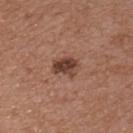Clinical impression:
Imaged during a routine full-body skin examination; the lesion was not biopsied and no histopathology is available.
Background:
Cropped from a total-body skin-imaging series; the visible field is about 15 mm. The subject is a male about 55 years old. An algorithmic analysis of the crop reported an outline eccentricity of about 0.75 (0 = round, 1 = elongated) and a symmetry-axis asymmetry near 0.2. The analysis additionally found an automated nevus-likeness rating near 80 out of 100 and lesion-presence confidence of about 100/100. The lesion's longest dimension is about 3.5 mm. The lesion is located on the chest. This is a white-light tile.